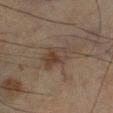Findings:
* biopsy status · imaged on a skin check; not biopsied
* subject · male, roughly 65 years of age
* illumination · cross-polarized
* lesion size · ~5 mm (longest diameter)
* imaging modality · ~15 mm crop, total-body skin-cancer survey
* site · the leg